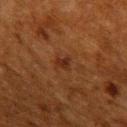No biopsy was performed on this lesion — it was imaged during a full skin examination and was not determined to be concerning.
A 15 mm close-up tile from a total-body photography series done for melanoma screening.
The patient is a male approximately 60 years of age.
The lesion's longest dimension is about 2.5 mm.
On the right upper arm.